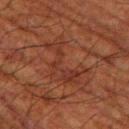{"biopsy_status": "not biopsied; imaged during a skin examination", "patient": {"sex": "male", "age_approx": 80}, "image": {"source": "total-body photography crop", "field_of_view_mm": 15}, "site": "left thigh", "lighting": "cross-polarized", "automated_metrics": {"area_mm2_approx": 11.0, "eccentricity": 0.85, "shape_asymmetry": 0.6, "color_variation_0_10": 2.5, "peripheral_color_asymmetry": 0.5, "nevus_likeness_0_100": 0, "lesion_detection_confidence_0_100": 75}}